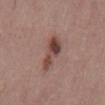• image-analysis metrics — an area of roughly 8.5 mm², a shape eccentricity near 0.9, and a shape-asymmetry score of about 0.4 (0 = symmetric); an automated nevus-likeness rating near 55 out of 100 and lesion-presence confidence of about 100/100
• body site — the abdomen
• acquisition — ~15 mm tile from a whole-body skin photo
• diameter — about 5 mm
• tile lighting — white-light illumination
• patient — male, aged 38–42